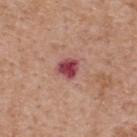biopsy_status: not biopsied; imaged during a skin examination
image:
  source: total-body photography crop
  field_of_view_mm: 15
lighting: white-light
patient:
  sex: male
  age_approx: 60
lesion_size:
  long_diameter_mm_approx: 2.5
site: upper back